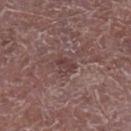Assessment:
Captured during whole-body skin photography for melanoma surveillance; the lesion was not biopsied.
Context:
A male subject aged around 65. A roughly 15 mm field-of-view crop from a total-body skin photograph. Automated image analysis of the tile measured about 8 CIELAB-L* units darker than the surrounding skin and a normalized lesion–skin contrast near 6.5. And it measured border irregularity of about 2.5 on a 0–10 scale, internal color variation of about 2.5 on a 0–10 scale, and peripheral color asymmetry of about 1. It also reported a nevus-likeness score of about 0/100 and a lesion-detection confidence of about 90/100. The tile uses white-light illumination. On the left lower leg. The lesion's longest dimension is about 2.5 mm.